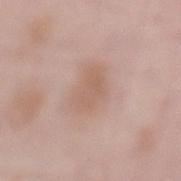Q: Was this lesion biopsied?
A: imaged on a skin check; not biopsied
Q: How large is the lesion?
A: about 4 mm
Q: What kind of image is this?
A: ~15 mm crop, total-body skin-cancer survey
Q: What are the patient's age and sex?
A: male, roughly 55 years of age
Q: Where on the body is the lesion?
A: the lower back